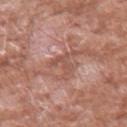Q: Who is the patient?
A: male, aged approximately 60
Q: How was the tile lit?
A: white-light illumination
Q: What kind of image is this?
A: 15 mm crop, total-body photography
Q: Lesion location?
A: the left forearm